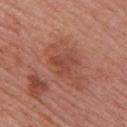Findings:
* biopsy status · catalogued during a skin exam; not biopsied
* body site · the right upper arm
* tile lighting · white-light
* automated lesion analysis · a footprint of about 5 mm² and a symmetry-axis asymmetry near 0.55; roughly 7 lightness units darker than nearby skin and a normalized lesion–skin contrast near 5.5; a within-lesion color-variation index near 1.5/10 and radial color variation of about 0
* subject · female, roughly 55 years of age
* lesion diameter · ≈3 mm
* acquisition · ~15 mm tile from a whole-body skin photo